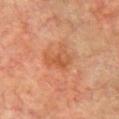This lesion was catalogued during total-body skin photography and was not selected for biopsy. Located on the chest. Captured under cross-polarized illumination. Automated image analysis of the tile measured a lesion area of about 6.5 mm², an eccentricity of roughly 0.65, and a symmetry-axis asymmetry near 0.4. It also reported an average lesion color of about L≈45 a*≈23 b*≈32 (CIELAB) and a lesion-to-skin contrast of about 6 (normalized; higher = more distinct). And it measured a classifier nevus-likeness of about 0/100. A male subject, aged 73 to 77. A 15 mm close-up tile from a total-body photography series done for melanoma screening.